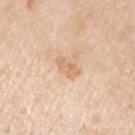Q: Was a biopsy performed?
A: total-body-photography surveillance lesion; no biopsy
Q: What is the imaging modality?
A: total-body-photography crop, ~15 mm field of view
Q: How was the tile lit?
A: white-light illumination
Q: Patient demographics?
A: male, aged 78 to 82
Q: How large is the lesion?
A: ≈3 mm
Q: Where on the body is the lesion?
A: the arm